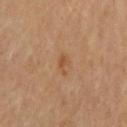Case summary:
* follow-up — total-body-photography surveillance lesion; no biopsy
* acquisition — 15 mm crop, total-body photography
* site — the chest
* subject — male, about 65 years old